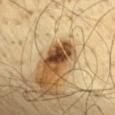Q: Is there a histopathology result?
A: total-body-photography surveillance lesion; no biopsy
Q: What is the imaging modality?
A: ~15 mm crop, total-body skin-cancer survey
Q: What is the lesion's diameter?
A: ≈4.5 mm
Q: How was the tile lit?
A: cross-polarized
Q: Lesion location?
A: the back
Q: What are the patient's age and sex?
A: male, roughly 60 years of age